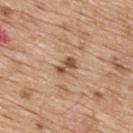This lesion was catalogued during total-body skin photography and was not selected for biopsy. A roughly 15 mm field-of-view crop from a total-body skin photograph. A male patient in their 70s. From the upper back. Imaged with white-light lighting. About 2.5 mm across. An algorithmic analysis of the crop reported a lesion area of about 4 mm², a shape eccentricity near 0.8, and two-axis asymmetry of about 0.25. The software also gave a lesion color around L≈53 a*≈20 b*≈31 in CIELAB and a lesion–skin lightness drop of about 12. It also reported a peripheral color-asymmetry measure near 2.